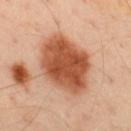Imaged during a routine full-body skin examination; the lesion was not biopsied and no histopathology is available. Captured under cross-polarized illumination. Automated image analysis of the tile measured border irregularity of about 2 on a 0–10 scale, a color-variation rating of about 5/10, and radial color variation of about 1.5. On the back. A roughly 15 mm field-of-view crop from a total-body skin photograph. The subject is a male in their mid-40s. About 7.5 mm across.